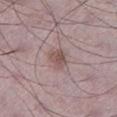biopsy status = catalogued during a skin exam; not biopsied | lighting = white-light illumination | subject = male, about 50 years old | image = total-body-photography crop, ~15 mm field of view | anatomic site = the left lower leg | automated metrics = a border-irregularity index near 2.5/10, a within-lesion color-variation index near 2.5/10, and a peripheral color-asymmetry measure near 1; a nevus-likeness score of about 85/100 | lesion diameter = about 3 mm.